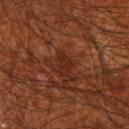Recorded during total-body skin imaging; not selected for excision or biopsy.
The lesion is located on the right upper arm.
A male subject, approximately 70 years of age.
A close-up tile cropped from a whole-body skin photograph, about 15 mm across.
Automated tile analysis of the lesion measured a border-irregularity rating of about 3/10, a color-variation rating of about 1/10, and peripheral color asymmetry of about 0.5.